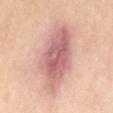Q: Is there a histopathology result?
A: imaged on a skin check; not biopsied
Q: Lesion location?
A: the abdomen
Q: What lighting was used for the tile?
A: cross-polarized illumination
Q: Who is the patient?
A: female, aged around 50
Q: What is the lesion's diameter?
A: ~8 mm (longest diameter)
Q: What is the imaging modality?
A: ~15 mm tile from a whole-body skin photo